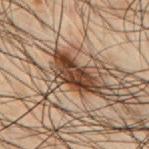biopsy status = catalogued during a skin exam; not biopsied | patient = male, approximately 50 years of age | imaging modality = ~15 mm crop, total-body skin-cancer survey | diameter = ~6 mm (longest diameter) | automated lesion analysis = a lesion area of about 10 mm², a shape eccentricity near 0.9, and two-axis asymmetry of about 0.3; a lesion color around L≈30 a*≈15 b*≈23 in CIELAB and roughly 14 lightness units darker than nearby skin; a border-irregularity rating of about 3.5/10 and internal color variation of about 5 on a 0–10 scale | location = the back.